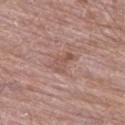{"biopsy_status": "not biopsied; imaged during a skin examination", "lesion_size": {"long_diameter_mm_approx": 3.0}, "patient": {"sex": "male", "age_approx": 65}, "image": {"source": "total-body photography crop", "field_of_view_mm": 15}, "automated_metrics": {"vs_skin_darker_L": 7.0, "vs_skin_contrast_norm": 5.5, "border_irregularity_0_10": 4.0, "color_variation_0_10": 2.0}, "site": "left thigh", "lighting": "white-light"}